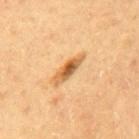{
  "biopsy_status": "not biopsied; imaged during a skin examination",
  "lighting": "cross-polarized",
  "lesion_size": {
    "long_diameter_mm_approx": 3.0
  },
  "image": {
    "source": "total-body photography crop",
    "field_of_view_mm": 15
  },
  "patient": {
    "sex": "male",
    "age_approx": 60
  },
  "site": "mid back"
}